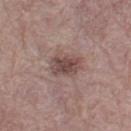The lesion was tiled from a total-body skin photograph and was not biopsied. The lesion is located on the left thigh. A roughly 15 mm field-of-view crop from a total-body skin photograph. Imaged with white-light lighting. A female patient, roughly 65 years of age. The recorded lesion diameter is about 4 mm.